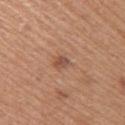Recorded during total-body skin imaging; not selected for excision or biopsy.
An algorithmic analysis of the crop reported a classifier nevus-likeness of about 5/100 and a lesion-detection confidence of about 100/100.
The patient is a female approximately 60 years of age.
This is a white-light tile.
The recorded lesion diameter is about 3.5 mm.
The lesion is on the right upper arm.
A 15 mm crop from a total-body photograph taken for skin-cancer surveillance.